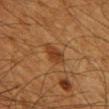The lesion was photographed on a routine skin check and not biopsied; there is no pathology result. The patient is a male approximately 60 years of age. The lesion's longest dimension is about 3 mm. From the right upper arm. Cropped from a whole-body photographic skin survey; the tile spans about 15 mm. The lesion-visualizer software estimated an area of roughly 5 mm², an eccentricity of roughly 0.7, and a symmetry-axis asymmetry near 0.25. The analysis additionally found a nevus-likeness score of about 75/100.